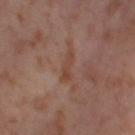Notes:
- follow-up: catalogued during a skin exam; not biopsied
- acquisition: ~15 mm crop, total-body skin-cancer survey
- patient: female, aged 53 to 57
- automated lesion analysis: a border-irregularity index near 4/10, a color-variation rating of about 0.5/10, and a peripheral color-asymmetry measure near 0
- site: the left thigh
- lighting: cross-polarized illumination